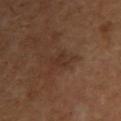{"biopsy_status": "not biopsied; imaged during a skin examination", "automated_metrics": {"area_mm2_approx": 3.0, "eccentricity": 0.7, "shape_asymmetry": 0.45, "border_irregularity_0_10": 4.5, "color_variation_0_10": 1.0, "peripheral_color_asymmetry": 0.0, "nevus_likeness_0_100": 0, "lesion_detection_confidence_0_100": 100}, "lighting": "cross-polarized", "patient": {"sex": "female", "age_approx": 50}, "image": {"source": "total-body photography crop", "field_of_view_mm": 15}, "site": "right upper arm", "lesion_size": {"long_diameter_mm_approx": 2.5}}